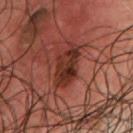notes: no biopsy performed (imaged during a skin exam)
subject: male, in their mid-60s
TBP lesion metrics: a lesion area of about 10 mm², an eccentricity of roughly 0.85, and a symmetry-axis asymmetry near 0.3; a mean CIELAB color near L≈29 a*≈24 b*≈25 and about 11 CIELAB-L* units darker than the surrounding skin
size: ≈5 mm
location: the front of the torso
image source: 15 mm crop, total-body photography